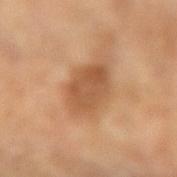{"biopsy_status": "not biopsied; imaged during a skin examination", "site": "left forearm", "lesion_size": {"long_diameter_mm_approx": 5.5}, "image": {"source": "total-body photography crop", "field_of_view_mm": 15}, "patient": {"sex": "male", "age_approx": 60}}